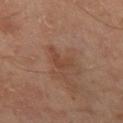biopsy_status: not biopsied; imaged during a skin examination
site: leg
automated_metrics:
  area_mm2_approx: 5.0
  eccentricity: 0.8
  shape_asymmetry: 0.55
  cielab_L: 42
  cielab_a: 20
  cielab_b: 28
  vs_skin_darker_L: 6.0
  vs_skin_contrast_norm: 5.5
  nevus_likeness_0_100: 0
  lesion_detection_confidence_0_100: 100
patient:
  sex: male
  age_approx: 70
image:
  source: total-body photography crop
  field_of_view_mm: 15